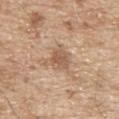No biopsy was performed on this lesion — it was imaged during a full skin examination and was not determined to be concerning. A male subject, about 70 years old. The recorded lesion diameter is about 4 mm. On the upper back. The total-body-photography lesion software estimated a lesion area of about 7.5 mm², a shape eccentricity near 0.6, and a symmetry-axis asymmetry near 0.35. And it measured border irregularity of about 4 on a 0–10 scale, a color-variation rating of about 2.5/10, and radial color variation of about 0.5. It also reported a lesion-detection confidence of about 100/100. Captured under white-light illumination. A region of skin cropped from a whole-body photographic capture, roughly 15 mm wide.